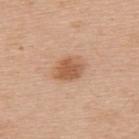follow-up = catalogued during a skin exam; not biopsied | lesion diameter = ~3.5 mm (longest diameter) | illumination = white-light illumination | automated lesion analysis = a footprint of about 7.5 mm², an eccentricity of roughly 0.65, and a symmetry-axis asymmetry near 0.2; internal color variation of about 3 on a 0–10 scale and a peripheral color-asymmetry measure near 1; an automated nevus-likeness rating near 95 out of 100 | acquisition = 15 mm crop, total-body photography | site = the upper back | patient = male, aged approximately 70.